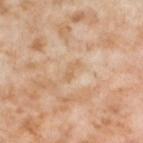Part of a total-body skin-imaging series; this lesion was reviewed on a skin check and was not flagged for biopsy. A 15 mm close-up tile from a total-body photography series done for melanoma screening. The subject is a female aged 53–57. The lesion is located on the left thigh.